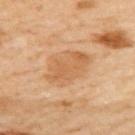Case summary:
- workup · imaged on a skin check; not biopsied
- anatomic site · the back
- automated metrics · a lesion area of about 15 mm², an outline eccentricity of about 0.7 (0 = round, 1 = elongated), and two-axis asymmetry of about 0.2; a lesion-to-skin contrast of about 5.5 (normalized; higher = more distinct); border irregularity of about 2.5 on a 0–10 scale, internal color variation of about 3 on a 0–10 scale, and radial color variation of about 1
- patient · female, aged 58–62
- illumination · cross-polarized
- lesion size · ≈5.5 mm
- acquisition · total-body-photography crop, ~15 mm field of view